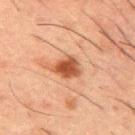Recorded during total-body skin imaging; not selected for excision or biopsy.
The tile uses cross-polarized illumination.
A male subject about 50 years old.
About 3 mm across.
A lesion tile, about 15 mm wide, cut from a 3D total-body photograph.
On the mid back.